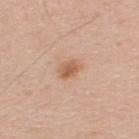Part of a total-body skin-imaging series; this lesion was reviewed on a skin check and was not flagged for biopsy. Measured at roughly 3 mm in maximum diameter. Automated image analysis of the tile measured an area of roughly 4.5 mm² and two-axis asymmetry of about 0.2. It also reported a mean CIELAB color near L≈60 a*≈21 b*≈33 and a normalized lesion–skin contrast near 7.5. A male patient, aged around 35. From the upper back. A 15 mm close-up extracted from a 3D total-body photography capture.